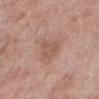The lesion was photographed on a routine skin check and not biopsied; there is no pathology result.
The lesion's longest dimension is about 3 mm.
This image is a 15 mm lesion crop taken from a total-body photograph.
Located on the left lower leg.
The lesion-visualizer software estimated an area of roughly 6.5 mm² and a symmetry-axis asymmetry near 0.35. And it measured a mean CIELAB color near L≈55 a*≈21 b*≈27, about 7 CIELAB-L* units darker than the surrounding skin, and a normalized border contrast of about 5. It also reported a classifier nevus-likeness of about 0/100.
The tile uses white-light illumination.
A female subject, aged approximately 70.